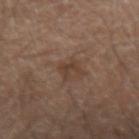Assessment: Captured during whole-body skin photography for melanoma surveillance; the lesion was not biopsied. Clinical summary: Located on the right forearm. Captured under cross-polarized illumination. The recorded lesion diameter is about 2.5 mm. Cropped from a whole-body photographic skin survey; the tile spans about 15 mm. Automated tile analysis of the lesion measured a classifier nevus-likeness of about 0/100 and a lesion-detection confidence of about 100/100. A male subject aged 63–67.